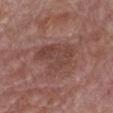biopsy_status: not biopsied; imaged during a skin examination
site: chest
lighting: white-light
patient:
  sex: male
  age_approx: 85+
image:
  source: total-body photography crop
  field_of_view_mm: 15
lesion_size:
  long_diameter_mm_approx: 5.5